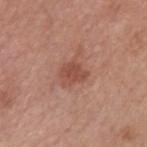Impression: No biopsy was performed on this lesion — it was imaged during a full skin examination and was not determined to be concerning. Clinical summary: A region of skin cropped from a whole-body photographic capture, roughly 15 mm wide. From the left upper arm. The lesion-visualizer software estimated an outline eccentricity of about 0.65 (0 = round, 1 = elongated) and a symmetry-axis asymmetry near 0.3. It also reported a classifier nevus-likeness of about 75/100 and a detector confidence of about 100 out of 100 that the crop contains a lesion. The patient is a male aged 53 to 57. The recorded lesion diameter is about 3 mm.